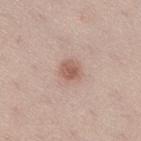subject = female, aged 38–42 | location = the right thigh | acquisition = ~15 mm crop, total-body skin-cancer survey | lesion size = about 2.5 mm.